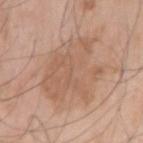Recorded during total-body skin imaging; not selected for excision or biopsy.
Approximately 7.5 mm at its widest.
An algorithmic analysis of the crop reported a border-irregularity rating of about 5.5/10 and peripheral color asymmetry of about 1. The analysis additionally found an automated nevus-likeness rating near 0 out of 100.
The subject is a male aged around 75.
A 15 mm close-up tile from a total-body photography series done for melanoma screening.
Located on the right upper arm.